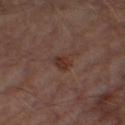Clinical impression:
Captured during whole-body skin photography for melanoma surveillance; the lesion was not biopsied.
Background:
Automated image analysis of the tile measured a mean CIELAB color near L≈30 a*≈18 b*≈22, roughly 7 lightness units darker than nearby skin, and a normalized lesion–skin contrast near 7.5. About 2.5 mm across. The lesion is on the right thigh. A 15 mm crop from a total-body photograph taken for skin-cancer surveillance. A male subject roughly 65 years of age.